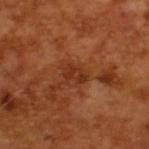biopsy status=imaged on a skin check; not biopsied | patient=male, roughly 65 years of age | image source=total-body-photography crop, ~15 mm field of view | diameter=about 2.5 mm.